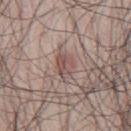Recorded during total-body skin imaging; not selected for excision or biopsy. The total-body-photography lesion software estimated a mean CIELAB color near L≈51 a*≈17 b*≈21, roughly 8 lightness units darker than nearby skin, and a normalized border contrast of about 6. Captured under white-light illumination. The lesion is located on the right thigh. Measured at roughly 3 mm in maximum diameter. A male subject aged 63–67. A lesion tile, about 15 mm wide, cut from a 3D total-body photograph.